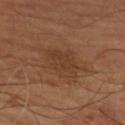Captured during whole-body skin photography for melanoma surveillance; the lesion was not biopsied. Approximately 4.5 mm at its widest. A lesion tile, about 15 mm wide, cut from a 3D total-body photograph. The lesion-visualizer software estimated a footprint of about 10 mm², an eccentricity of roughly 0.75, and two-axis asymmetry of about 0.2. The analysis additionally found an average lesion color of about L≈39 a*≈20 b*≈31 (CIELAB), a lesion–skin lightness drop of about 6, and a normalized lesion–skin contrast near 5. The software also gave internal color variation of about 2.5 on a 0–10 scale and peripheral color asymmetry of about 1. The software also gave a classifier nevus-likeness of about 0/100. Located on the arm. A male subject, roughly 65 years of age.